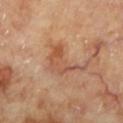<case>
  <biopsy_status>not biopsied; imaged during a skin examination</biopsy_status>
  <site>left lower leg</site>
  <image>
    <source>total-body photography crop</source>
    <field_of_view_mm>15</field_of_view_mm>
  </image>
  <automated_metrics>
    <vs_skin_darker_L>8.0</vs_skin_darker_L>
    <nevus_likeness_0_100>0</nevus_likeness_0_100>
    <lesion_detection_confidence_0_100>100</lesion_detection_confidence_0_100>
  </automated_metrics>
  <lighting>cross-polarized</lighting>
  <lesion_size>
    <long_diameter_mm_approx>5.5</long_diameter_mm_approx>
  </lesion_size>
  <patient>
    <sex>female</sex>
    <age_approx>60</age_approx>
  </patient>
</case>